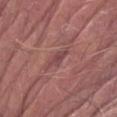Findings:
- body site: the left forearm
- patient: male, aged 73–77
- lighting: white-light illumination
- lesion size: about 2.5 mm
- TBP lesion metrics: an average lesion color of about L≈44 a*≈24 b*≈19 (CIELAB) and a normalized border contrast of about 6.5; an automated nevus-likeness rating near 0 out of 100 and lesion-presence confidence of about 60/100
- image: total-body-photography crop, ~15 mm field of view